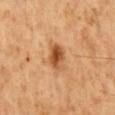Case summary:
• workup: no biopsy performed (imaged during a skin exam)
• subject: male, aged 58–62
• acquisition: 15 mm crop, total-body photography
• site: the back
• illumination: cross-polarized illumination